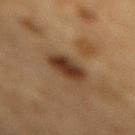<record>
  <biopsy_status>not biopsied; imaged during a skin examination</biopsy_status>
  <image>
    <source>total-body photography crop</source>
    <field_of_view_mm>15</field_of_view_mm>
  </image>
  <lighting>cross-polarized</lighting>
  <patient>
    <sex>male</sex>
    <age_approx>85+</age_approx>
  </patient>
  <lesion_size>
    <long_diameter_mm_approx>3.5</long_diameter_mm_approx>
  </lesion_size>
  <site>mid back</site>
</record>